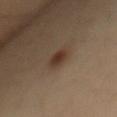A female patient in their mid-30s.
On the mid back.
A 15 mm close-up tile from a total-body photography series done for melanoma screening.
The recorded lesion diameter is about 3 mm.
Imaged with cross-polarized lighting.
Automated image analysis of the tile measured an area of roughly 4.5 mm², an outline eccentricity of about 0.8 (0 = round, 1 = elongated), and a shape-asymmetry score of about 0.2 (0 = symmetric).